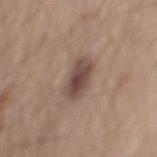  site: mid back
  image:
    source: total-body photography crop
    field_of_view_mm: 15
  patient:
    sex: male
    age_approx: 60